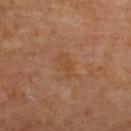Q: Was this lesion biopsied?
A: catalogued during a skin exam; not biopsied
Q: Illumination type?
A: cross-polarized illumination
Q: What are the patient's age and sex?
A: female, aged approximately 65
Q: What kind of image is this?
A: ~15 mm crop, total-body skin-cancer survey
Q: Automated lesion metrics?
A: a footprint of about 3.5 mm², a shape eccentricity near 0.9, and a symmetry-axis asymmetry near 0.3; an average lesion color of about L≈45 a*≈21 b*≈34 (CIELAB) and about 5 CIELAB-L* units darker than the surrounding skin; a border-irregularity rating of about 4/10, a within-lesion color-variation index near 1.5/10, and radial color variation of about 0.5; an automated nevus-likeness rating near 0 out of 100
Q: What is the anatomic site?
A: the back